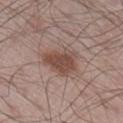This lesion was catalogued during total-body skin photography and was not selected for biopsy. Measured at roughly 4.5 mm in maximum diameter. A 15 mm crop from a total-body photograph taken for skin-cancer surveillance. The lesion is located on the right lower leg. Captured under white-light illumination. A male patient aged approximately 60.